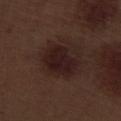<case>
<biopsy_status>not biopsied; imaged during a skin examination</biopsy_status>
<lighting>white-light</lighting>
<automated_metrics>
  <cielab_L>18</cielab_L>
  <cielab_a>17</cielab_a>
  <cielab_b>16</cielab_b>
  <vs_skin_darker_L>8.0</vs_skin_darker_L>
  <border_irregularity_0_10>2.0</border_irregularity_0_10>
  <color_variation_0_10>2.5</color_variation_0_10>
  <nevus_likeness_0_100>45</nevus_likeness_0_100>
  <lesion_detection_confidence_0_100>100</lesion_detection_confidence_0_100>
</automated_metrics>
<image>
  <source>total-body photography crop</source>
  <field_of_view_mm>15</field_of_view_mm>
</image>
<patient>
  <sex>male</sex>
  <age_approx>70</age_approx>
</patient>
<site>right lower leg</site>
</case>